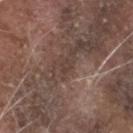Findings:
• workup: catalogued during a skin exam; not biopsied
• imaging modality: ~15 mm crop, total-body skin-cancer survey
• subject: male, in their mid-50s
• diameter: about 1.5 mm
• tile lighting: white-light illumination
• body site: the head or neck